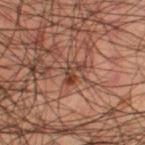Assessment:
The lesion was tiled from a total-body skin photograph and was not biopsied.
Context:
A male subject aged 48 to 52. On the leg. A close-up tile cropped from a whole-body skin photograph, about 15 mm across. Automated image analysis of the tile measured a lesion–skin lightness drop of about 8 and a normalized lesion–skin contrast near 7.5. The analysis additionally found a classifier nevus-likeness of about 0/100 and a lesion-detection confidence of about 85/100. Approximately 2.5 mm at its widest.